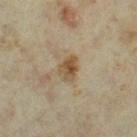The lesion was tiled from a total-body skin photograph and was not biopsied. The subject is a female aged approximately 35. Located on the left thigh. A close-up tile cropped from a whole-body skin photograph, about 15 mm across.